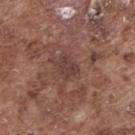- automated lesion analysis — a lesion area of about 5 mm² and two-axis asymmetry of about 0.3; a border-irregularity rating of about 2.5/10, internal color variation of about 1.5 on a 0–10 scale, and a peripheral color-asymmetry measure near 0.5
- patient — male, about 75 years old
- lighting — white-light illumination
- site — the back
- lesion size — ~3 mm (longest diameter)
- imaging modality — 15 mm crop, total-body photography